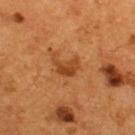Acquisition and patient details: Cropped from a total-body skin-imaging series; the visible field is about 15 mm. A male subject, in their mid-50s. The lesion is located on the back.